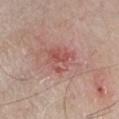acquisition: total-body-photography crop, ~15 mm field of view; subject: male, approximately 70 years of age; site: the chest.